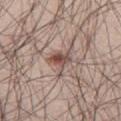* acquisition — total-body-photography crop, ~15 mm field of view
* anatomic site — the right thigh
* patient — male, aged around 30